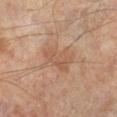No biopsy was performed on this lesion — it was imaged during a full skin examination and was not determined to be concerning. On the left lower leg. A male patient aged around 65. The tile uses cross-polarized illumination. Automated image analysis of the tile measured a normalized border contrast of about 5. And it measured border irregularity of about 3.5 on a 0–10 scale, internal color variation of about 2 on a 0–10 scale, and radial color variation of about 0.5. Cropped from a total-body skin-imaging series; the visible field is about 15 mm.